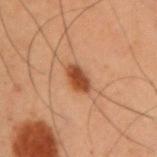Q: Is there a histopathology result?
A: imaged on a skin check; not biopsied
Q: Lesion size?
A: about 3 mm
Q: Where on the body is the lesion?
A: the right upper arm
Q: Patient demographics?
A: male, aged around 55
Q: What kind of image is this?
A: 15 mm crop, total-body photography
Q: Illumination type?
A: cross-polarized